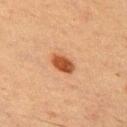* biopsy status — no biopsy performed (imaged during a skin exam)
* image — 15 mm crop, total-body photography
* illumination — cross-polarized illumination
* anatomic site — the front of the torso
* automated lesion analysis — border irregularity of about 2 on a 0–10 scale, a within-lesion color-variation index near 2.5/10, and a peripheral color-asymmetry measure near 1
* patient — male, aged approximately 50